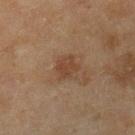<case>
<biopsy_status>not biopsied; imaged during a skin examination</biopsy_status>
<site>right lower leg</site>
<lesion_size>
  <long_diameter_mm_approx>2.5</long_diameter_mm_approx>
</lesion_size>
<lighting>cross-polarized</lighting>
<automated_metrics>
  <area_mm2_approx>5.0</area_mm2_approx>
  <eccentricity>0.2</eccentricity>
  <shape_asymmetry>0.15</shape_asymmetry>
  <nevus_likeness_0_100>5</nevus_likeness_0_100>
  <lesion_detection_confidence_0_100>100</lesion_detection_confidence_0_100>
</automated_metrics>
<image>
  <source>total-body photography crop</source>
  <field_of_view_mm>15</field_of_view_mm>
</image>
<patient>
  <sex>female</sex>
  <age_approx>60</age_approx>
</patient>
</case>